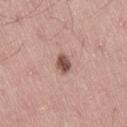Captured during whole-body skin photography for melanoma surveillance; the lesion was not biopsied.
From the leg.
An algorithmic analysis of the crop reported a footprint of about 3.5 mm², an eccentricity of roughly 0.75, and a shape-asymmetry score of about 0.35 (0 = symmetric). And it measured a lesion color around L≈51 a*≈22 b*≈23 in CIELAB, about 16 CIELAB-L* units darker than the surrounding skin, and a normalized lesion–skin contrast near 10.5. The software also gave border irregularity of about 3 on a 0–10 scale, a within-lesion color-variation index near 3/10, and peripheral color asymmetry of about 1.
A 15 mm close-up tile from a total-body photography series done for melanoma screening.
The patient is a male in their mid-40s.
Imaged with white-light lighting.
Longest diameter approximately 2.5 mm.